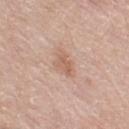Imaged during a routine full-body skin examination; the lesion was not biopsied and no histopathology is available. The subject is a male aged 78 to 82. A close-up tile cropped from a whole-body skin photograph, about 15 mm across. The lesion is located on the right thigh. About 3 mm across.